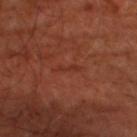Imaged during a routine full-body skin examination; the lesion was not biopsied and no histopathology is available.
Imaged with cross-polarized lighting.
The lesion is located on the leg.
The lesion's longest dimension is about 3 mm.
A region of skin cropped from a whole-body photographic capture, roughly 15 mm wide.
The total-body-photography lesion software estimated a footprint of about 2 mm² and two-axis asymmetry of about 0.4. The analysis additionally found a lesion–skin lightness drop of about 5 and a normalized border contrast of about 4.5. The software also gave a color-variation rating of about 0/10 and radial color variation of about 0. The software also gave an automated nevus-likeness rating near 0 out of 100.
The patient is in their mid-60s.